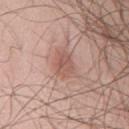<record>
  <biopsy_status>not biopsied; imaged during a skin examination</biopsy_status>
</record>